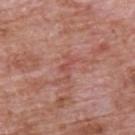biopsy status: imaged on a skin check; not biopsied
subject: male, aged approximately 70
image: 15 mm crop, total-body photography
lesion diameter: ~2.5 mm (longest diameter)
anatomic site: the upper back
tile lighting: white-light